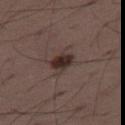diameter = ~3 mm (longest diameter) | imaging modality = ~15 mm tile from a whole-body skin photo | automated metrics = a border-irregularity index near 2.5/10, internal color variation of about 4 on a 0–10 scale, and radial color variation of about 1.5; a nevus-likeness score of about 90/100 and a detector confidence of about 100 out of 100 that the crop contains a lesion | body site = the right thigh | patient = male, in their 50s.